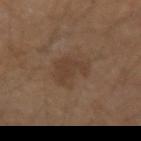{"biopsy_status": "not biopsied; imaged during a skin examination", "site": "arm", "patient": {"sex": "male", "age_approx": 40}, "image": {"source": "total-body photography crop", "field_of_view_mm": 15}}